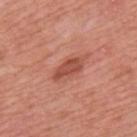| feature | finding |
|---|---|
| notes | total-body-photography surveillance lesion; no biopsy |
| size | ≈4 mm |
| body site | the mid back |
| subject | male, in their mid-60s |
| automated metrics | an area of roughly 5.5 mm², an eccentricity of roughly 0.9, and a symmetry-axis asymmetry near 0.25; a mean CIELAB color near L≈50 a*≈29 b*≈31, a lesion–skin lightness drop of about 11, and a normalized border contrast of about 7.5; a border-irregularity rating of about 3/10, internal color variation of about 2 on a 0–10 scale, and radial color variation of about 0.5 |
| imaging modality | total-body-photography crop, ~15 mm field of view |